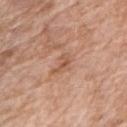The lesion was photographed on a routine skin check and not biopsied; there is no pathology result.
This image is a 15 mm lesion crop taken from a total-body photograph.
On the right upper arm.
A female patient aged around 75.